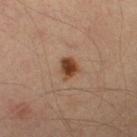Captured during whole-body skin photography for melanoma surveillance; the lesion was not biopsied.
A 15 mm close-up tile from a total-body photography series done for melanoma screening.
A patient about 55 years old.
On the right lower leg.
The recorded lesion diameter is about 2.5 mm.